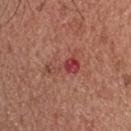* follow-up · catalogued during a skin exam; not biopsied
* image source · total-body-photography crop, ~15 mm field of view
* size · ≈4 mm
* patient · male, in their mid- to late 50s
* body site · the head or neck
* automated metrics · a footprint of about 5.5 mm², an eccentricity of roughly 0.9, and a symmetry-axis asymmetry near 0.65; an average lesion color of about L≈44 a*≈31 b*≈26 (CIELAB) and a lesion-to-skin contrast of about 7.5 (normalized; higher = more distinct); a border-irregularity rating of about 8/10, internal color variation of about 3 on a 0–10 scale, and a peripheral color-asymmetry measure near 1; a classifier nevus-likeness of about 0/100 and a detector confidence of about 100 out of 100 that the crop contains a lesion
* lighting · white-light illumination